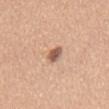Part of a total-body skin-imaging series; this lesion was reviewed on a skin check and was not flagged for biopsy. The lesion is on the chest. A female patient approximately 45 years of age. The recorded lesion diameter is about 2.5 mm. A 15 mm close-up tile from a total-body photography series done for melanoma screening.